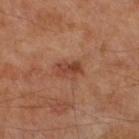This is a cross-polarized tile.
On the right lower leg.
An algorithmic analysis of the crop reported an area of roughly 4.5 mm², an outline eccentricity of about 0.85 (0 = round, 1 = elongated), and a symmetry-axis asymmetry near 0.2. The software also gave an average lesion color of about L≈41 a*≈25 b*≈30 (CIELAB). The analysis additionally found an automated nevus-likeness rating near 65 out of 100 and lesion-presence confidence of about 100/100.
A 15 mm close-up tile from a total-body photography series done for melanoma screening.
The patient is a male aged 68 to 72.